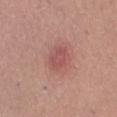biopsy status: imaged on a skin check; not biopsied
location: the left thigh
patient: female, aged around 50
size: about 3 mm
image: ~15 mm tile from a whole-body skin photo
automated metrics: a lesion area of about 5 mm², a shape eccentricity near 0.7, and two-axis asymmetry of about 0.3; a lesion color around L≈52 a*≈27 b*≈23 in CIELAB and a lesion-to-skin contrast of about 5.5 (normalized; higher = more distinct); border irregularity of about 3 on a 0–10 scale, internal color variation of about 1.5 on a 0–10 scale, and radial color variation of about 0.5; an automated nevus-likeness rating near 20 out of 100 and lesion-presence confidence of about 100/100
lighting: white-light illumination